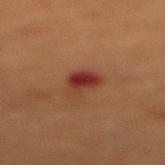This lesion was catalogued during total-body skin photography and was not selected for biopsy. The tile uses cross-polarized illumination. Cropped from a total-body skin-imaging series; the visible field is about 15 mm. Automated image analysis of the tile measured about 8 CIELAB-L* units darker than the surrounding skin and a normalized border contrast of about 9. The software also gave a classifier nevus-likeness of about 0/100 and a lesion-detection confidence of about 100/100. A female subject, aged 78–82. The lesion is located on the chest.